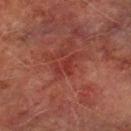notes = no biopsy performed (imaged during a skin exam) | image source = ~15 mm tile from a whole-body skin photo | tile lighting = cross-polarized illumination | lesion diameter = ~3.5 mm (longest diameter) | patient = male, aged 73–77 | body site = the left lower leg | image-analysis metrics = a lesion area of about 4 mm², a shape eccentricity near 0.8, and two-axis asymmetry of about 0.55; a nevus-likeness score of about 0/100 and lesion-presence confidence of about 70/100.